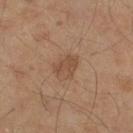Case summary:
- follow-up · no biopsy performed (imaged during a skin exam)
- image · ~15 mm crop, total-body skin-cancer survey
- tile lighting · cross-polarized
- patient · male, about 60 years old
- size · about 3 mm
- site · the left thigh